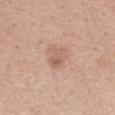notes = total-body-photography surveillance lesion; no biopsy
acquisition = ~15 mm tile from a whole-body skin photo
location = the left forearm
patient = female, aged around 30